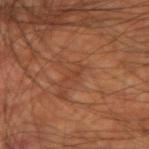biopsy_status: not biopsied; imaged during a skin examination
lesion_size:
  long_diameter_mm_approx: 2.5
automated_metrics:
  nevus_likeness_0_100: 0
  lesion_detection_confidence_0_100: 85
lighting: cross-polarized
patient:
  sex: male
  age_approx: 60
site: right upper arm
image:
  source: total-body photography crop
  field_of_view_mm: 15